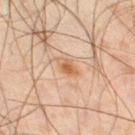Impression: Imaged during a routine full-body skin examination; the lesion was not biopsied and no histopathology is available. Context: The lesion is on the right thigh. A male subject, roughly 45 years of age. About 2.5 mm across. Cropped from a whole-body photographic skin survey; the tile spans about 15 mm. This is a cross-polarized tile.